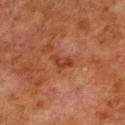The lesion was photographed on a routine skin check and not biopsied; there is no pathology result.
Imaged with cross-polarized lighting.
About 2.5 mm across.
A male subject aged approximately 80.
A 15 mm crop from a total-body photograph taken for skin-cancer surveillance.
Automated tile analysis of the lesion measured an area of roughly 2.5 mm² and an outline eccentricity of about 0.85 (0 = round, 1 = elongated). And it measured internal color variation of about 0 on a 0–10 scale and radial color variation of about 0.
From the right upper arm.